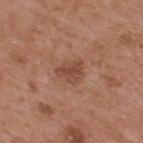Recorded during total-body skin imaging; not selected for excision or biopsy.
Longest diameter approximately 3 mm.
The total-body-photography lesion software estimated a lesion area of about 6 mm², an outline eccentricity of about 0.6 (0 = round, 1 = elongated), and a symmetry-axis asymmetry near 0.35. It also reported a mean CIELAB color near L≈47 a*≈22 b*≈29 and a lesion-to-skin contrast of about 6.5 (normalized; higher = more distinct). It also reported border irregularity of about 3.5 on a 0–10 scale, internal color variation of about 2 on a 0–10 scale, and radial color variation of about 0.5. And it measured an automated nevus-likeness rating near 10 out of 100 and a lesion-detection confidence of about 100/100.
The subject is a male aged 53 to 57.
The lesion is on the upper back.
A 15 mm crop from a total-body photograph taken for skin-cancer surveillance.